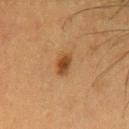Clinical impression:
Captured during whole-body skin photography for melanoma surveillance; the lesion was not biopsied.
Clinical summary:
The recorded lesion diameter is about 3 mm. Captured under cross-polarized illumination. The lesion is on the chest. Automated tile analysis of the lesion measured a mean CIELAB color near L≈37 a*≈19 b*≈32, roughly 11 lightness units darker than nearby skin, and a lesion-to-skin contrast of about 9.5 (normalized; higher = more distinct). A 15 mm close-up tile from a total-body photography series done for melanoma screening. The patient is a female aged 48 to 52.